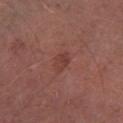Findings:
– biopsy status — imaged on a skin check; not biopsied